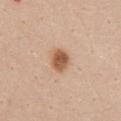The lesion was photographed on a routine skin check and not biopsied; there is no pathology result. A 15 mm crop from a total-body photograph taken for skin-cancer surveillance. The tile uses white-light illumination. The lesion is located on the abdomen. An algorithmic analysis of the crop reported a mean CIELAB color near L≈57 a*≈22 b*≈33, a lesion–skin lightness drop of about 14, and a normalized lesion–skin contrast near 9.5. And it measured a border-irregularity rating of about 2/10 and a color-variation rating of about 3.5/10. It also reported a detector confidence of about 100 out of 100 that the crop contains a lesion. A female subject, roughly 40 years of age.